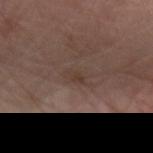Captured during whole-body skin photography for melanoma surveillance; the lesion was not biopsied.
Captured under white-light illumination.
A male subject, aged 63–67.
Located on the left forearm.
A 15 mm close-up tile from a total-body photography series done for melanoma screening.
Longest diameter approximately 2.5 mm.